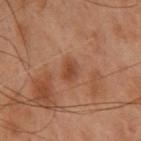biopsy status = total-body-photography surveillance lesion; no biopsy | automated lesion analysis = an area of roughly 4 mm², an eccentricity of roughly 0.7, and two-axis asymmetry of about 0.2; border irregularity of about 2 on a 0–10 scale, a color-variation rating of about 2/10, and a peripheral color-asymmetry measure near 0.5 | image source = total-body-photography crop, ~15 mm field of view | subject = female, in their mid-50s | diameter = about 2.5 mm | body site = the upper back.